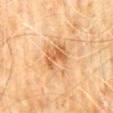follow-up = no biopsy performed (imaged during a skin exam)
imaging modality = ~15 mm crop, total-body skin-cancer survey
tile lighting = cross-polarized illumination
lesion size = ~3.5 mm (longest diameter)
anatomic site = the abdomen
subject = male, aged 58–62
automated lesion analysis = a mean CIELAB color near L≈61 a*≈24 b*≈42, roughly 11 lightness units darker than nearby skin, and a normalized lesion–skin contrast near 7; a border-irregularity index near 4.5/10 and peripheral color asymmetry of about 1.5; a classifier nevus-likeness of about 25/100